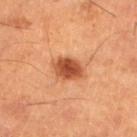The lesion was tiled from a total-body skin photograph and was not biopsied. A male patient, aged 58–62. This image is a 15 mm lesion crop taken from a total-body photograph. On the leg. Imaged with cross-polarized lighting.